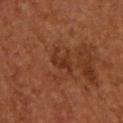biopsy_status: not biopsied; imaged during a skin examination
site: upper back
patient:
  sex: male
  age_approx: 55
image:
  source: total-body photography crop
  field_of_view_mm: 15